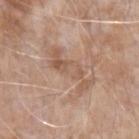Longest diameter approximately 6 mm.
The tile uses white-light illumination.
A male subject, approximately 75 years of age.
This image is a 15 mm lesion crop taken from a total-body photograph.
Located on the left forearm.
An algorithmic analysis of the crop reported a lesion area of about 11 mm², an outline eccentricity of about 0.95 (0 = round, 1 = elongated), and a shape-asymmetry score of about 0.45 (0 = symmetric). It also reported a border-irregularity index near 8.5/10, internal color variation of about 3.5 on a 0–10 scale, and radial color variation of about 1. And it measured lesion-presence confidence of about 95/100.